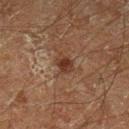location: the left lower leg
size: ~2.5 mm (longest diameter)
image: ~15 mm crop, total-body skin-cancer survey
subject: male, aged 58 to 62